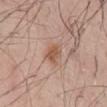<case>
  <biopsy_status>not biopsied; imaged during a skin examination</biopsy_status>
  <patient>
    <sex>male</sex>
    <age_approx>60</age_approx>
  </patient>
  <image>
    <source>total-body photography crop</source>
    <field_of_view_mm>15</field_of_view_mm>
  </image>
  <site>front of the torso</site>
</case>